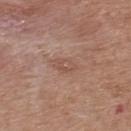imaging modality=~15 mm tile from a whole-body skin photo; subject=male, aged approximately 55; body site=the upper back.